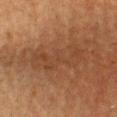Notes:
- notes: total-body-photography surveillance lesion; no biopsy
- anatomic site: the chest
- illumination: cross-polarized illumination
- subject: male, roughly 65 years of age
- imaging modality: ~15 mm crop, total-body skin-cancer survey
- diameter: ~7 mm (longest diameter)